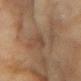Part of a total-body skin-imaging series; this lesion was reviewed on a skin check and was not flagged for biopsy.